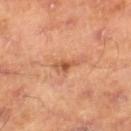Background:
Approximately 3 mm at its widest. A 15 mm close-up tile from a total-body photography series done for melanoma screening. The subject is a male aged approximately 45. On the right lower leg. This is a cross-polarized tile.